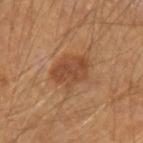Case summary:
• biopsy status — total-body-photography surveillance lesion; no biopsy
• tile lighting — cross-polarized illumination
• patient — male, in their mid- to late 60s
• image source — ~15 mm tile from a whole-body skin photo
• lesion diameter — about 4 mm
• location — the left forearm